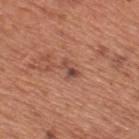{"biopsy_status": "not biopsied; imaged during a skin examination", "site": "upper back", "lesion_size": {"long_diameter_mm_approx": 2.5}, "patient": {"sex": "male", "age_approx": 65}, "image": {"source": "total-body photography crop", "field_of_view_mm": 15}}